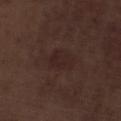  biopsy_status: not biopsied; imaged during a skin examination
  patient:
    sex: male
    age_approx: 70
  site: leg
  image:
    source: total-body photography crop
    field_of_view_mm: 15
  automated_metrics:
    border_irregularity_0_10: 1.5
    color_variation_0_10: 2.0
    peripheral_color_asymmetry: 0.5
    nevus_likeness_0_100: 15
    lesion_detection_confidence_0_100: 100